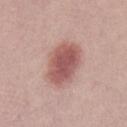<case>
<automated_metrics>
  <area_mm2_approx>16.0</area_mm2_approx>
  <eccentricity>0.8</eccentricity>
  <shape_asymmetry>0.15</shape_asymmetry>
  <cielab_L>55</cielab_L>
  <cielab_a>24</cielab_a>
  <cielab_b>22</cielab_b>
  <vs_skin_darker_L>14.0</vs_skin_darker_L>
  <vs_skin_contrast_norm>9.0</vs_skin_contrast_norm>
  <color_variation_0_10>3.5</color_variation_0_10>
  <peripheral_color_asymmetry>1.0</peripheral_color_asymmetry>
  <nevus_likeness_0_100>100</nevus_likeness_0_100>
  <lesion_detection_confidence_0_100>100</lesion_detection_confidence_0_100>
</automated_metrics>
<patient>
  <sex>male</sex>
  <age_approx>30</age_approx>
</patient>
<lighting>white-light</lighting>
<image>
  <source>total-body photography crop</source>
  <field_of_view_mm>15</field_of_view_mm>
</image>
<lesion_size>
  <long_diameter_mm_approx>5.5</long_diameter_mm_approx>
</lesion_size>
</case>